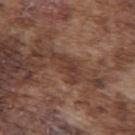Case summary:
- notes · no biopsy performed (imaged during a skin exam)
- acquisition · 15 mm crop, total-body photography
- TBP lesion metrics · an area of roughly 5.5 mm² and a shape eccentricity near 0.9; a border-irregularity rating of about 6.5/10
- patient · male, about 75 years old
- lesion size · ~4.5 mm (longest diameter)
- location · the upper back